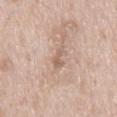{"site": "mid back", "lighting": "white-light", "lesion_size": {"long_diameter_mm_approx": 2.5}, "image": {"source": "total-body photography crop", "field_of_view_mm": 15}, "patient": {"sex": "male", "age_approx": 65}}